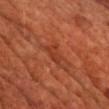Part of a total-body skin-imaging series; this lesion was reviewed on a skin check and was not flagged for biopsy. Longest diameter approximately 2.5 mm. The lesion is on the chest. A male patient, approximately 70 years of age. Imaged with cross-polarized lighting. Cropped from a whole-body photographic skin survey; the tile spans about 15 mm.